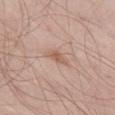Imaged during a routine full-body skin examination; the lesion was not biopsied and no histopathology is available.
A 15 mm crop from a total-body photograph taken for skin-cancer surveillance.
Imaged with white-light lighting.
From the left thigh.
A male subject, in their mid- to late 50s.